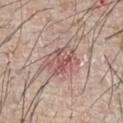Case summary:
– workup · imaged on a skin check; not biopsied
– patient · male, in their mid-60s
– image · ~15 mm tile from a whole-body skin photo
– location · the front of the torso